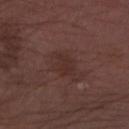{
  "biopsy_status": "not biopsied; imaged during a skin examination",
  "site": "left forearm",
  "image": {
    "source": "total-body photography crop",
    "field_of_view_mm": 15
  },
  "automated_metrics": {
    "area_mm2_approx": 5.5,
    "shape_asymmetry": 0.25,
    "cielab_L": 29,
    "cielab_a": 18,
    "cielab_b": 20,
    "vs_skin_contrast_norm": 5.5,
    "border_irregularity_0_10": 2.5,
    "color_variation_0_10": 2.0
  },
  "patient": {
    "sex": "male",
    "age_approx": 75
  },
  "lesion_size": {
    "long_diameter_mm_approx": 3.5
  }
}